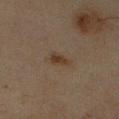workup — total-body-photography surveillance lesion; no biopsy | tile lighting — cross-polarized | acquisition — ~15 mm crop, total-body skin-cancer survey | site — the right upper arm | patient — male, in their mid-60s.